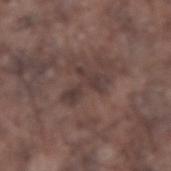  biopsy_status: not biopsied; imaged during a skin examination